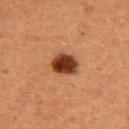Part of a total-body skin-imaging series; this lesion was reviewed on a skin check and was not flagged for biopsy.
The tile uses cross-polarized illumination.
Cropped from a total-body skin-imaging series; the visible field is about 15 mm.
A female patient aged approximately 40.
The lesion is located on the upper back.
The lesion-visualizer software estimated an automated nevus-likeness rating near 100 out of 100 and lesion-presence confidence of about 100/100.
About 3.5 mm across.